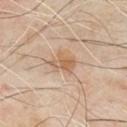follow-up: catalogued during a skin exam; not biopsied | anatomic site: the front of the torso | patient: male, about 50 years old | imaging modality: 15 mm crop, total-body photography | tile lighting: cross-polarized.